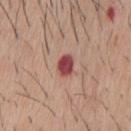Part of a total-body skin-imaging series; this lesion was reviewed on a skin check and was not flagged for biopsy. This image is a 15 mm lesion crop taken from a total-body photograph. A male subject, aged approximately 60. The recorded lesion diameter is about 2.5 mm. Captured under white-light illumination. The total-body-photography lesion software estimated a lesion area of about 4 mm², an eccentricity of roughly 0.7, and a shape-asymmetry score of about 0.15 (0 = symmetric). And it measured a lesion color around L≈46 a*≈32 b*≈23 in CIELAB. The software also gave a border-irregularity index near 1.5/10, a color-variation rating of about 4/10, and a peripheral color-asymmetry measure near 1.5. From the chest.